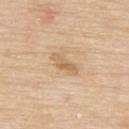Captured during whole-body skin photography for melanoma surveillance; the lesion was not biopsied.
Captured under white-light illumination.
On the back.
A female subject, roughly 70 years of age.
Cropped from a total-body skin-imaging series; the visible field is about 15 mm.
Longest diameter approximately 3.5 mm.